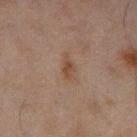Recorded during total-body skin imaging; not selected for excision or biopsy. The patient is a male aged around 45. The lesion is located on the leg. A region of skin cropped from a whole-body photographic capture, roughly 15 mm wide.